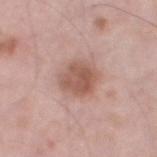| key | value |
|---|---|
| notes | imaged on a skin check; not biopsied |
| image source | total-body-photography crop, ~15 mm field of view |
| diameter | ~4 mm (longest diameter) |
| anatomic site | the left thigh |
| patient | male, aged around 55 |
| tile lighting | white-light illumination |